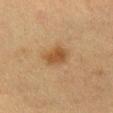Q: Is there a histopathology result?
A: total-body-photography surveillance lesion; no biopsy
Q: How large is the lesion?
A: about 3.5 mm
Q: Automated lesion metrics?
A: a lesion area of about 6 mm², an eccentricity of roughly 0.75, and a symmetry-axis asymmetry near 0.2; a mean CIELAB color near L≈41 a*≈17 b*≈33, a lesion–skin lightness drop of about 9, and a normalized lesion–skin contrast near 8; a border-irregularity index near 2/10 and a peripheral color-asymmetry measure near 0.5
Q: Patient demographics?
A: female, in their mid- to late 50s
Q: What kind of image is this?
A: total-body-photography crop, ~15 mm field of view
Q: How was the tile lit?
A: cross-polarized illumination
Q: What is the anatomic site?
A: the leg